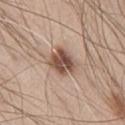Case summary:
* notes · total-body-photography surveillance lesion; no biopsy
* diameter · ≈3.5 mm
* tile lighting · white-light
* imaging modality · 15 mm crop, total-body photography
* location · the chest
* patient · male, aged 43–47
* automated lesion analysis · an outline eccentricity of about 0.6 (0 = round, 1 = elongated) and two-axis asymmetry of about 0.25; a border-irregularity rating of about 2.5/10, a within-lesion color-variation index near 5.5/10, and peripheral color asymmetry of about 2; an automated nevus-likeness rating near 70 out of 100 and lesion-presence confidence of about 100/100